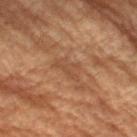site = the right forearm
tile lighting = cross-polarized
image-analysis metrics = a mean CIELAB color near L≈35 a*≈16 b*≈25; border irregularity of about 4 on a 0–10 scale, a color-variation rating of about 1/10, and radial color variation of about 0.5; a lesion-detection confidence of about 85/100
lesion diameter = ~3.5 mm (longest diameter)
imaging modality = total-body-photography crop, ~15 mm field of view
subject = female, aged 78 to 82